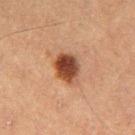Q: Was this lesion biopsied?
A: no biopsy performed (imaged during a skin exam)
Q: Where on the body is the lesion?
A: the leg
Q: What did automated image analysis measure?
A: border irregularity of about 1.5 on a 0–10 scale, a within-lesion color-variation index near 4.5/10, and a peripheral color-asymmetry measure near 1.5; an automated nevus-likeness rating near 100 out of 100 and lesion-presence confidence of about 100/100
Q: What are the patient's age and sex?
A: female, in their 60s
Q: Lesion size?
A: about 3.5 mm
Q: What kind of image is this?
A: 15 mm crop, total-body photography